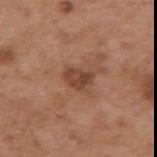Captured during whole-body skin photography for melanoma surveillance; the lesion was not biopsied. A 15 mm close-up tile from a total-body photography series done for melanoma screening. A male subject roughly 55 years of age. Longest diameter approximately 3 mm. The lesion is located on the right upper arm. The total-body-photography lesion software estimated roughly 10 lightness units darker than nearby skin. The analysis additionally found a classifier nevus-likeness of about 25/100 and a detector confidence of about 100 out of 100 that the crop contains a lesion.